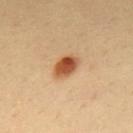Impression:
Imaged during a routine full-body skin examination; the lesion was not biopsied and no histopathology is available.
Image and clinical context:
A roughly 15 mm field-of-view crop from a total-body skin photograph. The lesion is on the back. Longest diameter approximately 3 mm. The subject is a male approximately 40 years of age. This is a cross-polarized tile.